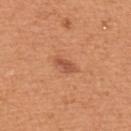Assessment: The lesion was photographed on a routine skin check and not biopsied; there is no pathology result. Background: Measured at roughly 2.5 mm in maximum diameter. The total-body-photography lesion software estimated an automated nevus-likeness rating near 90 out of 100. A male patient in their mid- to late 50s. On the upper back. A roughly 15 mm field-of-view crop from a total-body skin photograph. Captured under white-light illumination.